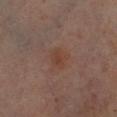The lesion was tiled from a total-body skin photograph and was not biopsied. A male subject roughly 60 years of age. Captured under cross-polarized illumination. A region of skin cropped from a whole-body photographic capture, roughly 15 mm wide. Measured at roughly 2.5 mm in maximum diameter. The lesion is located on the left lower leg.